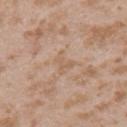Q: Is there a histopathology result?
A: catalogued during a skin exam; not biopsied
Q: How was the tile lit?
A: white-light
Q: What are the patient's age and sex?
A: female, approximately 25 years of age
Q: What is the anatomic site?
A: the left upper arm
Q: What kind of image is this?
A: 15 mm crop, total-body photography
Q: Lesion size?
A: ~3 mm (longest diameter)